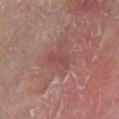Impression:
The lesion was tiled from a total-body skin photograph and was not biopsied.